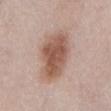- follow-up: no biopsy performed (imaged during a skin exam)
- acquisition: total-body-photography crop, ~15 mm field of view
- site: the abdomen
- patient: female, in their 50s